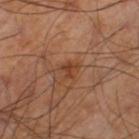Q: How was this image acquired?
A: 15 mm crop, total-body photography
Q: Illumination type?
A: cross-polarized
Q: What is the anatomic site?
A: the leg
Q: What are the patient's age and sex?
A: male, in their 60s
Q: Lesion size?
A: about 2 mm